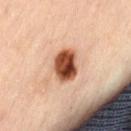Q: What did automated image analysis measure?
A: border irregularity of about 1.5 on a 0–10 scale, internal color variation of about 9 on a 0–10 scale, and radial color variation of about 3.5
Q: How was this image acquired?
A: ~15 mm tile from a whole-body skin photo
Q: How large is the lesion?
A: ~4 mm (longest diameter)
Q: Patient demographics?
A: female, aged 58–62
Q: What lighting was used for the tile?
A: cross-polarized illumination
Q: Lesion location?
A: the lower back